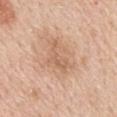Q: Is there a histopathology result?
A: no biopsy performed (imaged during a skin exam)
Q: What kind of image is this?
A: total-body-photography crop, ~15 mm field of view
Q: What is the anatomic site?
A: the mid back
Q: What are the patient's age and sex?
A: male, aged 58–62